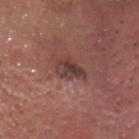| field | value |
|---|---|
| follow-up | no biopsy performed (imaged during a skin exam) |
| subject | male, about 55 years old |
| lesion size | ≈3.5 mm |
| acquisition | 15 mm crop, total-body photography |
| site | the head or neck |
| lighting | white-light |
| automated metrics | a shape eccentricity near 0.65; a lesion color around L≈39 a*≈21 b*≈21 in CIELAB, a lesion–skin lightness drop of about 9, and a normalized lesion–skin contrast near 7.5; border irregularity of about 3 on a 0–10 scale and internal color variation of about 5.5 on a 0–10 scale; an automated nevus-likeness rating near 5 out of 100 and lesion-presence confidence of about 100/100 |